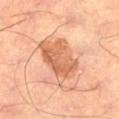notes = total-body-photography surveillance lesion; no biopsy | site = the left thigh | subject = male, about 65 years old | diameter = about 6 mm | illumination = cross-polarized illumination | image source = ~15 mm tile from a whole-body skin photo.